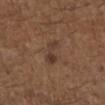Q: Is there a histopathology result?
A: no biopsy performed (imaged during a skin exam)